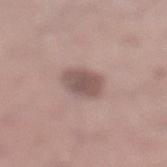The lesion was photographed on a routine skin check and not biopsied; there is no pathology result. A 15 mm close-up extracted from a 3D total-body photography capture. On the leg. A male subject, about 35 years old.